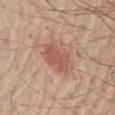{
  "biopsy_status": "not biopsied; imaged during a skin examination",
  "patient": {
    "sex": "male",
    "age_approx": 70
  },
  "image": {
    "source": "total-body photography crop",
    "field_of_view_mm": 15
  },
  "site": "chest"
}